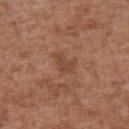<record>
  <biopsy_status>not biopsied; imaged during a skin examination</biopsy_status>
  <image>
    <source>total-body photography crop</source>
    <field_of_view_mm>15</field_of_view_mm>
  </image>
  <automated_metrics>
    <area_mm2_approx>3.0</area_mm2_approx>
    <shape_asymmetry>0.5</shape_asymmetry>
    <cielab_L>44</cielab_L>
    <cielab_a>22</cielab_a>
    <cielab_b>30</cielab_b>
    <vs_skin_darker_L>7.0</vs_skin_darker_L>
    <border_irregularity_0_10>4.5</border_irregularity_0_10>
    <peripheral_color_asymmetry>0.0</peripheral_color_asymmetry>
  </automated_metrics>
  <lighting>white-light</lighting>
  <site>left upper arm</site>
  <lesion_size>
    <long_diameter_mm_approx>2.5</long_diameter_mm_approx>
  </lesion_size>
  <patient>
    <sex>male</sex>
    <age_approx>75</age_approx>
  </patient>
</record>